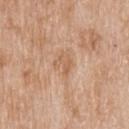| key | value |
|---|---|
| follow-up | catalogued during a skin exam; not biopsied |
| diameter | about 3 mm |
| anatomic site | the upper back |
| image | ~15 mm crop, total-body skin-cancer survey |
| TBP lesion metrics | a border-irregularity rating of about 5/10, internal color variation of about 2 on a 0–10 scale, and radial color variation of about 0.5 |
| subject | male, aged 78 to 82 |
| illumination | white-light |